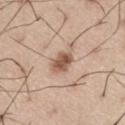Recorded during total-body skin imaging; not selected for excision or biopsy.
The patient is a male aged 53–57.
A 15 mm close-up extracted from a 3D total-body photography capture.
From the right thigh.